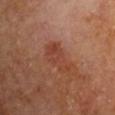Context: A close-up tile cropped from a whole-body skin photograph, about 15 mm across. An algorithmic analysis of the crop reported a footprint of about 7.5 mm² and two-axis asymmetry of about 0.45. The lesion's longest dimension is about 4.5 mm. Imaged with cross-polarized lighting. Located on the right upper arm. The subject is a male roughly 70 years of age.